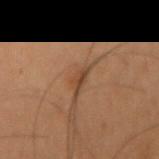The lesion was photographed on a routine skin check and not biopsied; there is no pathology result. The lesion is located on the right upper arm. A male patient in their mid-50s. A 15 mm close-up extracted from a 3D total-body photography capture.